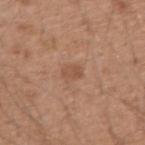The lesion was tiled from a total-body skin photograph and was not biopsied.
A male patient, aged around 50.
On the right upper arm.
A lesion tile, about 15 mm wide, cut from a 3D total-body photograph.